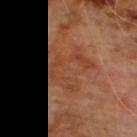<case>
  <biopsy_status>not biopsied; imaged during a skin examination</biopsy_status>
  <image>
    <source>total-body photography crop</source>
    <field_of_view_mm>15</field_of_view_mm>
  </image>
  <automated_metrics>
    <area_mm2_approx>17.0</area_mm2_approx>
    <eccentricity>0.5</eccentricity>
    <shape_asymmetry>0.45</shape_asymmetry>
    <border_irregularity_0_10>6.0</border_irregularity_0_10>
    <color_variation_0_10>5.0</color_variation_0_10>
    <peripheral_color_asymmetry>1.5</peripheral_color_asymmetry>
  </automated_metrics>
  <lighting>cross-polarized</lighting>
  <patient>
    <sex>male</sex>
    <age_approx>60</age_approx>
  </patient>
  <site>chest</site>
  <lesion_size>
    <long_diameter_mm_approx>5.5</long_diameter_mm_approx>
  </lesion_size>
</case>